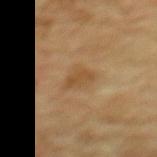The lesion was tiled from a total-body skin photograph and was not biopsied.
The lesion is on the upper back.
Automated tile analysis of the lesion measured a mean CIELAB color near L≈46 a*≈17 b*≈35, roughly 7 lightness units darker than nearby skin, and a normalized border contrast of about 6.
A male patient, approximately 70 years of age.
Cropped from a total-body skin-imaging series; the visible field is about 15 mm.
Imaged with cross-polarized lighting.